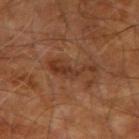Assessment: Captured during whole-body skin photography for melanoma surveillance; the lesion was not biopsied. Acquisition and patient details: Captured under cross-polarized illumination. The patient is a male aged around 70. Cropped from a whole-body photographic skin survey; the tile spans about 15 mm. From the right upper arm. The total-body-photography lesion software estimated a border-irregularity rating of about 9.5/10, a within-lesion color-variation index near 2.5/10, and radial color variation of about 0.5.